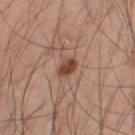Clinical impression:
Recorded during total-body skin imaging; not selected for excision or biopsy.
Background:
A 15 mm close-up extracted from a 3D total-body photography capture. A male patient roughly 50 years of age. Located on the abdomen.